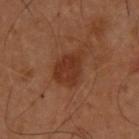Q: Is there a histopathology result?
A: no biopsy performed (imaged during a skin exam)
Q: What is the imaging modality?
A: ~15 mm tile from a whole-body skin photo
Q: Who is the patient?
A: male, approximately 55 years of age
Q: Lesion location?
A: the upper back